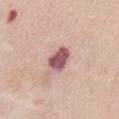The lesion was photographed on a routine skin check and not biopsied; there is no pathology result. Automated image analysis of the tile measured an outline eccentricity of about 0.75 (0 = round, 1 = elongated) and a shape-asymmetry score of about 0.2 (0 = symmetric). And it measured an average lesion color of about L≈54 a*≈26 b*≈17 (CIELAB), about 18 CIELAB-L* units darker than the surrounding skin, and a normalized lesion–skin contrast near 12. It also reported a nevus-likeness score of about 40/100. A 15 mm close-up extracted from a 3D total-body photography capture. Measured at roughly 3.5 mm in maximum diameter. Imaged with white-light lighting. The lesion is on the chest. A female patient, roughly 65 years of age.